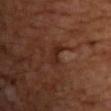Captured during whole-body skin photography for melanoma surveillance; the lesion was not biopsied. A roughly 15 mm field-of-view crop from a total-body skin photograph. The recorded lesion diameter is about 3 mm. A male subject, approximately 55 years of age. On the front of the torso. Imaged with cross-polarized lighting. The total-body-photography lesion software estimated a footprint of about 4.5 mm², a shape eccentricity near 0.85, and a shape-asymmetry score of about 0.4 (0 = symmetric). The analysis additionally found a border-irregularity index near 4/10, internal color variation of about 1.5 on a 0–10 scale, and peripheral color asymmetry of about 0.5. It also reported a nevus-likeness score of about 0/100 and lesion-presence confidence of about 75/100.